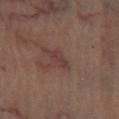Recorded during total-body skin imaging; not selected for excision or biopsy. An algorithmic analysis of the crop reported a border-irregularity index near 4/10, a color-variation rating of about 0/10, and peripheral color asymmetry of about 0. Located on the left lower leg. Approximately 3 mm at its widest. A 15 mm crop from a total-body photograph taken for skin-cancer surveillance. A subject aged approximately 65. Imaged with cross-polarized lighting.